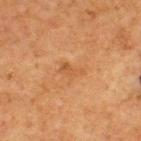Q: Was a biopsy performed?
A: imaged on a skin check; not biopsied
Q: Illumination type?
A: cross-polarized illumination
Q: What is the imaging modality?
A: total-body-photography crop, ~15 mm field of view
Q: Lesion size?
A: ≈3 mm
Q: Who is the patient?
A: male, aged 73–77
Q: Where on the body is the lesion?
A: the back